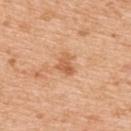{
  "biopsy_status": "not biopsied; imaged during a skin examination",
  "lesion_size": {
    "long_diameter_mm_approx": 2.5
  },
  "lighting": "white-light",
  "image": {
    "source": "total-body photography crop",
    "field_of_view_mm": 15
  },
  "patient": {
    "sex": "male",
    "age_approx": 60
  },
  "automated_metrics": {
    "area_mm2_approx": 4.0,
    "eccentricity": 0.65,
    "shape_asymmetry": 0.5
  },
  "site": "upper back"
}